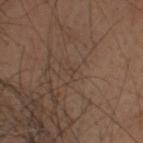Recorded during total-body skin imaging; not selected for excision or biopsy. From the head or neck. The patient is a male about 55 years old. A lesion tile, about 15 mm wide, cut from a 3D total-body photograph.